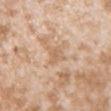Part of a total-body skin-imaging series; this lesion was reviewed on a skin check and was not flagged for biopsy.
Captured under white-light illumination.
Measured at roughly 3 mm in maximum diameter.
A female subject aged approximately 25.
Located on the left upper arm.
A 15 mm close-up extracted from a 3D total-body photography capture.